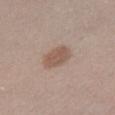The lesion was photographed on a routine skin check and not biopsied; there is no pathology result. A 15 mm close-up tile from a total-body photography series done for melanoma screening. The recorded lesion diameter is about 3.5 mm. The lesion is on the leg. The patient is a female about 20 years old. Imaged with white-light lighting.